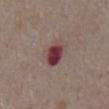tile lighting: white-light illumination; body site: the abdomen; subject: male, aged 73–77; image source: total-body-photography crop, ~15 mm field of view.